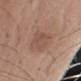| key | value |
|---|---|
| location | the arm |
| diameter | ~5.5 mm (longest diameter) |
| acquisition | ~15 mm crop, total-body skin-cancer survey |
| subject | male, aged 68 to 72 |